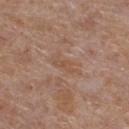patient:
  sex: female
  age_approx: 55
lighting: white-light
site: left lower leg
image:
  source: total-body photography crop
  field_of_view_mm: 15